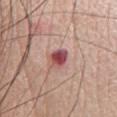{"biopsy_status": "not biopsied; imaged during a skin examination", "image": {"source": "total-body photography crop", "field_of_view_mm": 15}, "patient": {"sex": "male", "age_approx": 65}, "site": "chest", "lesion_size": {"long_diameter_mm_approx": 3.0}, "lighting": "white-light"}